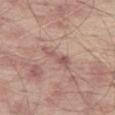follow-up = imaged on a skin check; not biopsied
patient = male, about 65 years old
image = ~15 mm tile from a whole-body skin photo
anatomic site = the left thigh
automated lesion analysis = a lesion area of about 5 mm² and two-axis asymmetry of about 0.45; a lesion–skin lightness drop of about 9; a lesion-detection confidence of about 100/100